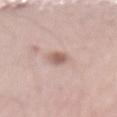No biopsy was performed on this lesion — it was imaged during a full skin examination and was not determined to be concerning.
Located on the back.
A male patient, approximately 65 years of age.
The tile uses white-light illumination.
A close-up tile cropped from a whole-body skin photograph, about 15 mm across.